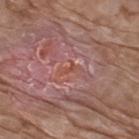Q: What did the biopsy show?
A: a nodular basal cell carcinoma — a malignant lesion